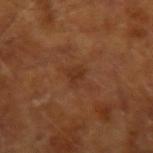Assessment:
The lesion was tiled from a total-body skin photograph and was not biopsied.
Acquisition and patient details:
Imaged with cross-polarized lighting. This image is a 15 mm lesion crop taken from a total-body photograph. Located on the left forearm. Measured at roughly 4 mm in maximum diameter. A male subject about 65 years old.